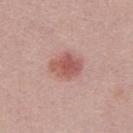| feature | finding |
|---|---|
| follow-up | no biopsy performed (imaged during a skin exam) |
| image source | 15 mm crop, total-body photography |
| subject | male, roughly 30 years of age |
| image-analysis metrics | a lesion color around L≈55 a*≈25 b*≈25 in CIELAB |
| diameter | about 4 mm |
| illumination | white-light |